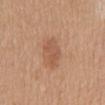Impression: Imaged during a routine full-body skin examination; the lesion was not biopsied and no histopathology is available. Context: A 15 mm close-up extracted from a 3D total-body photography capture. The lesion's longest dimension is about 3.5 mm. The lesion is on the back. Imaged with white-light lighting. A female subject aged approximately 55.